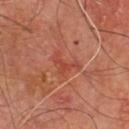The lesion was tiled from a total-body skin photograph and was not biopsied. The lesion's longest dimension is about 3.5 mm. The tile uses cross-polarized illumination. A male subject, about 65 years old. Located on the chest. A 15 mm crop from a total-body photograph taken for skin-cancer surveillance. Automated tile analysis of the lesion measured an area of roughly 4.5 mm², a shape eccentricity near 0.8, and a shape-asymmetry score of about 0.65 (0 = symmetric). The software also gave a border-irregularity rating of about 7.5/10, a within-lesion color-variation index near 1.5/10, and radial color variation of about 0.5. The software also gave an automated nevus-likeness rating near 0 out of 100 and a detector confidence of about 95 out of 100 that the crop contains a lesion.